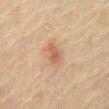notes=catalogued during a skin exam; not biopsied | diameter=≈2.5 mm | illumination=cross-polarized illumination | patient=male, roughly 70 years of age | location=the front of the torso | image=~15 mm crop, total-body skin-cancer survey.